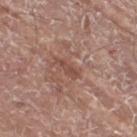Imaged during a routine full-body skin examination; the lesion was not biopsied and no histopathology is available. Cropped from a total-body skin-imaging series; the visible field is about 15 mm. The tile uses white-light illumination. The recorded lesion diameter is about 3 mm. Located on the right thigh. An algorithmic analysis of the crop reported a lesion area of about 3 mm². The analysis additionally found an average lesion color of about L≈48 a*≈22 b*≈26 (CIELAB), a lesion–skin lightness drop of about 7, and a normalized lesion–skin contrast near 6. The software also gave a within-lesion color-variation index near 0.5/10 and radial color variation of about 0. The software also gave an automated nevus-likeness rating near 0 out of 100 and a lesion-detection confidence of about 95/100. A male subject aged 73 to 77.